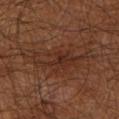workup: catalogued during a skin exam; not biopsied
subject: male, about 45 years old
body site: the right upper arm
automated metrics: a lesion color around L≈28 a*≈19 b*≈25 in CIELAB and a lesion-to-skin contrast of about 6 (normalized; higher = more distinct); a classifier nevus-likeness of about 0/100 and lesion-presence confidence of about 100/100
lesion size: about 7.5 mm
illumination: cross-polarized illumination
imaging modality: total-body-photography crop, ~15 mm field of view